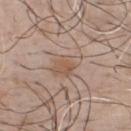{"patient": {"sex": "male", "age_approx": 45}, "site": "front of the torso", "image": {"source": "total-body photography crop", "field_of_view_mm": 15}}